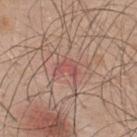{"biopsy_status": "not biopsied; imaged during a skin examination", "patient": {"sex": "male", "age_approx": 50}, "image": {"source": "total-body photography crop", "field_of_view_mm": 15}, "site": "upper back"}